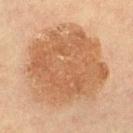{
  "biopsy_status": "not biopsied; imaged during a skin examination",
  "site": "leg",
  "patient": {
    "sex": "female",
    "age_approx": 65
  },
  "lighting": "cross-polarized",
  "image": {
    "source": "total-body photography crop",
    "field_of_view_mm": 15
  }
}